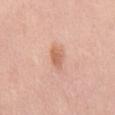image = total-body-photography crop, ~15 mm field of view | site = the mid back | subject = female, about 40 years old.